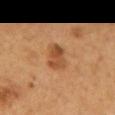Clinical impression: No biopsy was performed on this lesion — it was imaged during a full skin examination and was not determined to be concerning. Clinical summary: Longest diameter approximately 4 mm. On the back. A lesion tile, about 15 mm wide, cut from a 3D total-body photograph. Captured under cross-polarized illumination. The lesion-visualizer software estimated a lesion area of about 6.5 mm², an eccentricity of roughly 0.9, and two-axis asymmetry of about 0.25. The software also gave border irregularity of about 2.5 on a 0–10 scale. The patient is a male aged 53–57.